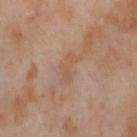Findings:
- biopsy status · catalogued during a skin exam; not biopsied
- TBP lesion metrics · a lesion area of about 4.5 mm² and a shape-asymmetry score of about 0.4 (0 = symmetric); an average lesion color of about L≈55 a*≈18 b*≈30 (CIELAB), roughly 5 lightness units darker than nearby skin, and a normalized lesion–skin contrast near 4.5; a border-irregularity index near 5/10, a within-lesion color-variation index near 1/10, and peripheral color asymmetry of about 0
- site · the right thigh
- acquisition · total-body-photography crop, ~15 mm field of view
- illumination · cross-polarized
- lesion size · ≈3.5 mm
- patient · female, in their mid-50s